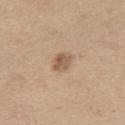The lesion was photographed on a routine skin check and not biopsied; there is no pathology result.
The lesion is on the leg.
A male patient aged approximately 65.
A 15 mm crop from a total-body photograph taken for skin-cancer surveillance.
Approximately 2.5 mm at its widest.
This is a white-light tile.